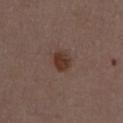A male subject about 50 years old. Cropped from a total-body skin-imaging series; the visible field is about 15 mm. The lesion is on the abdomen. The tile uses white-light illumination.